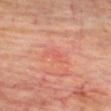<lesion>
  <biopsy_status>not biopsied; imaged during a skin examination</biopsy_status>
  <patient>
    <sex>male</sex>
    <age_approx>60</age_approx>
  </patient>
  <lighting>cross-polarized</lighting>
  <lesion_size>
    <long_diameter_mm_approx>2.5</long_diameter_mm_approx>
  </lesion_size>
  <automated_metrics>
    <area_mm2_approx>3.0</area_mm2_approx>
    <eccentricity>0.8</eccentricity>
    <shape_asymmetry>0.55</shape_asymmetry>
  </automated_metrics>
  <image>
    <source>total-body photography crop</source>
    <field_of_view_mm>15</field_of_view_mm>
  </image>
  <site>chest</site>
</lesion>